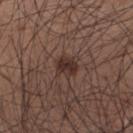<lesion>
  <biopsy_status>not biopsied; imaged during a skin examination</biopsy_status>
  <patient>
    <sex>male</sex>
    <age_approx>35</age_approx>
  </patient>
  <site>mid back</site>
  <lighting>white-light</lighting>
  <image>
    <source>total-body photography crop</source>
    <field_of_view_mm>15</field_of_view_mm>
  </image>
</lesion>